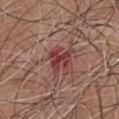Assessment:
Recorded during total-body skin imaging; not selected for excision or biopsy.
Background:
A 15 mm crop from a total-body photograph taken for skin-cancer surveillance. About 3.5 mm across. A male patient aged 73 to 77. From the chest. This is a white-light tile.